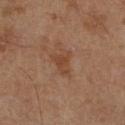Context:
A 15 mm close-up extracted from a 3D total-body photography capture. This is a cross-polarized tile. Automated tile analysis of the lesion measured a footprint of about 4.5 mm² and a symmetry-axis asymmetry near 0.55. The software also gave a classifier nevus-likeness of about 0/100. A male subject, approximately 65 years of age. The lesion's longest dimension is about 3 mm. The lesion is located on the right lower leg.